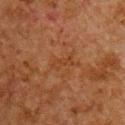No biopsy was performed on this lesion — it was imaged during a full skin examination and was not determined to be concerning. On the front of the torso. A male patient, approximately 60 years of age. This image is a 15 mm lesion crop taken from a total-body photograph.